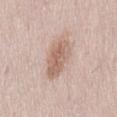| key | value |
|---|---|
| imaging modality | ~15 mm tile from a whole-body skin photo |
| subject | female, aged approximately 30 |
| size | ~5.5 mm (longest diameter) |
| location | the back |
| automated metrics | a color-variation rating of about 4/10 and a peripheral color-asymmetry measure near 1.5; lesion-presence confidence of about 100/100 |
| illumination | white-light |